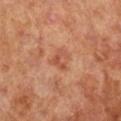<tbp_lesion>
  <biopsy_status>not biopsied; imaged during a skin examination</biopsy_status>
  <lesion_size>
    <long_diameter_mm_approx>2.5</long_diameter_mm_approx>
  </lesion_size>
  <image>
    <source>total-body photography crop</source>
    <field_of_view_mm>15</field_of_view_mm>
  </image>
  <patient>
    <sex>female</sex>
    <age_approx>65</age_approx>
  </patient>
  <site>right lower leg</site>
</tbp_lesion>